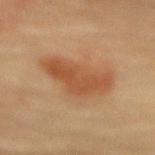Cropped from a whole-body photographic skin survey; the tile spans about 15 mm. A female subject about 80 years old. About 6.5 mm across. The lesion is on the back.